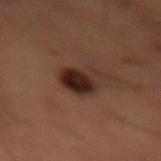<lesion>
  <biopsy_status>not biopsied; imaged during a skin examination</biopsy_status>
  <automated_metrics>
    <area_mm2_approx>9.5</area_mm2_approx>
    <eccentricity>0.55</eccentricity>
    <shape_asymmetry>0.3</shape_asymmetry>
    <border_irregularity_0_10>3.0</border_irregularity_0_10>
    <color_variation_0_10>7.0</color_variation_0_10>
    <peripheral_color_asymmetry>2.5</peripheral_color_asymmetry>
    <nevus_likeness_0_100>90</nevus_likeness_0_100>
    <lesion_detection_confidence_0_100>100</lesion_detection_confidence_0_100>
  </automated_metrics>
  <site>lower back</site>
  <image>
    <source>total-body photography crop</source>
    <field_of_view_mm>15</field_of_view_mm>
  </image>
  <lighting>cross-polarized</lighting>
  <lesion_size>
    <long_diameter_mm_approx>4.0</long_diameter_mm_approx>
  </lesion_size>
  <patient>
    <sex>male</sex>
    <age_approx>50</age_approx>
  </patient>
</lesion>